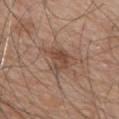Clinical impression:
The lesion was tiled from a total-body skin photograph and was not biopsied.
Background:
The lesion is on the chest. A male patient aged 68 to 72. Longest diameter approximately 3.5 mm. A region of skin cropped from a whole-body photographic capture, roughly 15 mm wide.